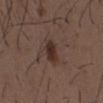Image and clinical context: A male subject, aged 48–52. On the mid back. Measured at roughly 3.5 mm in maximum diameter. Captured under white-light illumination. A lesion tile, about 15 mm wide, cut from a 3D total-body photograph.